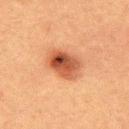Imaged during a routine full-body skin examination; the lesion was not biopsied and no histopathology is available.
A 15 mm close-up tile from a total-body photography series done for melanoma screening.
From the upper back.
A male patient, aged 38–42.
This is a cross-polarized tile.
Automated image analysis of the tile measured a footprint of about 10 mm² and a symmetry-axis asymmetry near 0.2. It also reported a lesion–skin lightness drop of about 13. It also reported a nevus-likeness score of about 100/100 and lesion-presence confidence of about 100/100.
Approximately 4 mm at its widest.